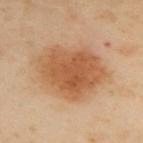Q: Was this lesion biopsied?
A: total-body-photography surveillance lesion; no biopsy
Q: How was the tile lit?
A: cross-polarized
Q: Lesion location?
A: the upper back
Q: Automated lesion metrics?
A: a lesion area of about 31 mm², an outline eccentricity of about 0.8 (0 = round, 1 = elongated), and a symmetry-axis asymmetry near 0.2; a mean CIELAB color near L≈48 a*≈20 b*≈33 and a normalized border contrast of about 8.5; a border-irregularity rating of about 2/10, internal color variation of about 3.5 on a 0–10 scale, and peripheral color asymmetry of about 1; a nevus-likeness score of about 100/100 and a detector confidence of about 100 out of 100 that the crop contains a lesion
Q: How was this image acquired?
A: total-body-photography crop, ~15 mm field of view
Q: What are the patient's age and sex?
A: female, aged 58–62
Q: What is the lesion's diameter?
A: ~8.5 mm (longest diameter)